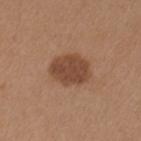biopsy status=imaged on a skin check; not biopsied | diameter=≈4.5 mm | anatomic site=the arm | image=~15 mm crop, total-body skin-cancer survey | subject=female, aged approximately 40.